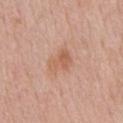The lesion was tiled from a total-body skin photograph and was not biopsied.
The subject is a male aged 58–62.
The lesion is on the front of the torso.
Cropped from a total-body skin-imaging series; the visible field is about 15 mm.